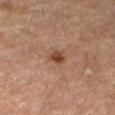Notes:
- biopsy status: imaged on a skin check; not biopsied
- anatomic site: the right thigh
- image-analysis metrics: a footprint of about 3.5 mm², a shape eccentricity near 0.8, and a shape-asymmetry score of about 0.35 (0 = symmetric)
- size: ≈2.5 mm
- imaging modality: 15 mm crop, total-body photography
- illumination: cross-polarized illumination
- subject: female, aged 53–57